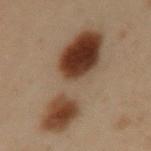notes: total-body-photography surveillance lesion; no biopsy | imaging modality: 15 mm crop, total-body photography | image-analysis metrics: a border-irregularity index near 4.5/10, a within-lesion color-variation index near 10/10, and a peripheral color-asymmetry measure near 5.5 | site: the left upper arm | lesion size: ≈11 mm | subject: male, in their mid- to late 50s.